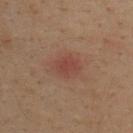Impression: No biopsy was performed on this lesion — it was imaged during a full skin examination and was not determined to be concerning. Background: The recorded lesion diameter is about 3.5 mm. A male patient, in their mid- to late 30s. A lesion tile, about 15 mm wide, cut from a 3D total-body photograph. The lesion is on the upper back.